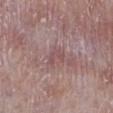| feature | finding |
|---|---|
| biopsy status | total-body-photography surveillance lesion; no biopsy |
| diameter | about 2.5 mm |
| illumination | white-light |
| image-analysis metrics | a mean CIELAB color near L≈50 a*≈21 b*≈18, a lesion–skin lightness drop of about 7, and a lesion-to-skin contrast of about 5 (normalized; higher = more distinct) |
| patient | male, approximately 75 years of age |
| acquisition | ~15 mm crop, total-body skin-cancer survey |
| body site | the leg |